A male patient, aged 53–57 · on the mid back · a lesion tile, about 15 mm wide, cut from a 3D total-body photograph:
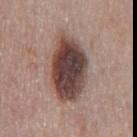About 7.5 mm across. Imaged with white-light lighting. Automated image analysis of the tile measured an area of roughly 25 mm², a shape eccentricity near 0.8, and a shape-asymmetry score of about 0.15 (0 = symmetric). It also reported a nevus-likeness score of about 75/100 and lesion-presence confidence of about 100/100. On excision, pathology confirmed a dysplastic (Clark) nevus, classified as a benign lesion.